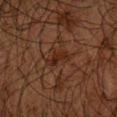Imaged during a routine full-body skin examination; the lesion was not biopsied and no histopathology is available. A male subject aged around 65. This is a cross-polarized tile. The lesion is located on the right forearm. A roughly 15 mm field-of-view crop from a total-body skin photograph. Approximately 3 mm at its widest. Automated image analysis of the tile measured a shape eccentricity near 0.85 and a shape-asymmetry score of about 0.45 (0 = symmetric).